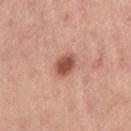Clinical impression:
The lesion was tiled from a total-body skin photograph and was not biopsied.
Clinical summary:
The lesion is on the left thigh. Longest diameter approximately 3 mm. A female subject approximately 25 years of age. Automated image analysis of the tile measured a mean CIELAB color near L≈53 a*≈26 b*≈29, roughly 14 lightness units darker than nearby skin, and a lesion-to-skin contrast of about 9.5 (normalized; higher = more distinct). And it measured a within-lesion color-variation index near 3/10 and peripheral color asymmetry of about 1. A roughly 15 mm field-of-view crop from a total-body skin photograph. Imaged with white-light lighting.